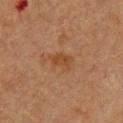Findings:
- notes — imaged on a skin check; not biopsied
- location — the chest
- acquisition — ~15 mm tile from a whole-body skin photo
- patient — female, roughly 40 years of age
- tile lighting — cross-polarized